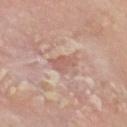biopsy status: catalogued during a skin exam; not biopsied | tile lighting: white-light illumination | TBP lesion metrics: a border-irregularity index near 3/10 and internal color variation of about 2 on a 0–10 scale | anatomic site: the head or neck | subject: male, roughly 80 years of age | lesion size: ≈3 mm | acquisition: total-body-photography crop, ~15 mm field of view.